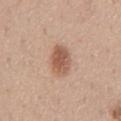No biopsy was performed on this lesion — it was imaged during a full skin examination and was not determined to be concerning.
From the abdomen.
A male patient, roughly 55 years of age.
Automated tile analysis of the lesion measured an area of roughly 9 mm², a shape eccentricity near 0.85, and two-axis asymmetry of about 0.1. The software also gave a border-irregularity index near 1.5/10, internal color variation of about 4.5 on a 0–10 scale, and radial color variation of about 1.5.
A region of skin cropped from a whole-body photographic capture, roughly 15 mm wide.
Measured at roughly 4.5 mm in maximum diameter.